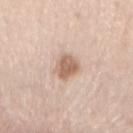This lesion was catalogued during total-body skin photography and was not selected for biopsy. A 15 mm close-up tile from a total-body photography series done for melanoma screening. On the chest. Imaged with white-light lighting. The lesion's longest dimension is about 4 mm. Automated image analysis of the tile measured a border-irregularity rating of about 2.5/10, a color-variation rating of about 2.5/10, and radial color variation of about 1. And it measured a nevus-likeness score of about 70/100 and lesion-presence confidence of about 100/100. A female subject, aged 53–57.